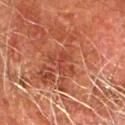Q: Was this lesion biopsied?
A: imaged on a skin check; not biopsied
Q: What kind of image is this?
A: ~15 mm crop, total-body skin-cancer survey
Q: Lesion location?
A: the right forearm
Q: Illumination type?
A: cross-polarized
Q: How large is the lesion?
A: ~3.5 mm (longest diameter)
Q: Who is the patient?
A: male, roughly 80 years of age